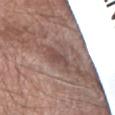– follow-up: total-body-photography surveillance lesion; no biopsy
– image: total-body-photography crop, ~15 mm field of view
– diameter: ≈3 mm
– site: the right forearm
– subject: male, aged around 70